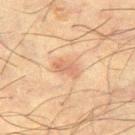{
  "biopsy_status": "not biopsied; imaged during a skin examination",
  "lighting": "cross-polarized",
  "lesion_size": {
    "long_diameter_mm_approx": 3.5
  },
  "site": "left thigh",
  "image": {
    "source": "total-body photography crop",
    "field_of_view_mm": 15
  },
  "patient": {
    "sex": "male",
    "age_approx": 65
  }
}